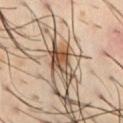notes — imaged on a skin check; not biopsied
diameter — about 5.5 mm
acquisition — 15 mm crop, total-body photography
subject — male, aged around 40
location — the front of the torso
illumination — cross-polarized